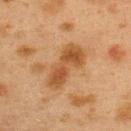Impression:
The lesion was photographed on a routine skin check and not biopsied; there is no pathology result.
Acquisition and patient details:
A 15 mm crop from a total-body photograph taken for skin-cancer surveillance. The lesion is on the back. The subject is a female in their 40s.